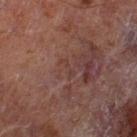Clinical impression:
The lesion was tiled from a total-body skin photograph and was not biopsied.
Context:
Approximately 2.5 mm at its widest. The total-body-photography lesion software estimated an area of roughly 2 mm², an outline eccentricity of about 0.95 (0 = round, 1 = elongated), and a symmetry-axis asymmetry near 0.4. The software also gave an average lesion color of about L≈30 a*≈16 b*≈19 (CIELAB) and a lesion-to-skin contrast of about 4.5 (normalized; higher = more distinct). The analysis additionally found a border-irregularity rating of about 6/10. The software also gave a nevus-likeness score of about 0/100 and a lesion-detection confidence of about 55/100. The tile uses cross-polarized illumination. A male patient, aged approximately 70. A region of skin cropped from a whole-body photographic capture, roughly 15 mm wide. On the leg.